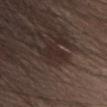Q: Was this lesion biopsied?
A: no biopsy performed (imaged during a skin exam)
Q: Where on the body is the lesion?
A: the head or neck
Q: Patient demographics?
A: female, aged approximately 35
Q: What lighting was used for the tile?
A: white-light illumination
Q: How was this image acquired?
A: 15 mm crop, total-body photography
Q: Lesion size?
A: about 3 mm